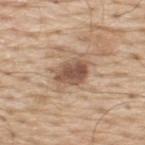| feature | finding |
|---|---|
| workup | imaged on a skin check; not biopsied |
| illumination | white-light |
| site | the upper back |
| patient | male, roughly 70 years of age |
| acquisition | 15 mm crop, total-body photography |